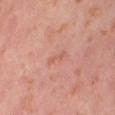| feature | finding |
|---|---|
| workup | no biopsy performed (imaged during a skin exam) |